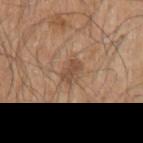{"biopsy_status": "not biopsied; imaged during a skin examination", "site": "arm", "lighting": "white-light", "lesion_size": {"long_diameter_mm_approx": 3.0}, "patient": {"sex": "male", "age_approx": 80}, "image": {"source": "total-body photography crop", "field_of_view_mm": 15}, "automated_metrics": {"area_mm2_approx": 4.5, "eccentricity": 0.75, "cielab_L": 49, "cielab_a": 18, "cielab_b": 30, "vs_skin_darker_L": 9.0, "vs_skin_contrast_norm": 6.5, "nevus_likeness_0_100": 30, "lesion_detection_confidence_0_100": 100}}